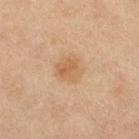Assessment:
Imaged during a routine full-body skin examination; the lesion was not biopsied and no histopathology is available.